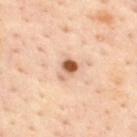Q: Is there a histopathology result?
A: imaged on a skin check; not biopsied
Q: Lesion location?
A: the upper back
Q: How was this image acquired?
A: total-body-photography crop, ~15 mm field of view
Q: How large is the lesion?
A: about 3 mm
Q: Automated lesion metrics?
A: an area of roughly 4.5 mm², a shape eccentricity near 0.75, and a symmetry-axis asymmetry near 0.4; a lesion-detection confidence of about 100/100
Q: What are the patient's age and sex?
A: male, aged approximately 45